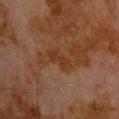Captured during whole-body skin photography for melanoma surveillance; the lesion was not biopsied.
The recorded lesion diameter is about 3 mm.
The subject is a male approximately 80 years of age.
Cropped from a total-body skin-imaging series; the visible field is about 15 mm.
Imaged with cross-polarized lighting.
Located on the upper back.
An algorithmic analysis of the crop reported a mean CIELAB color near L≈26 a*≈17 b*≈27 and a normalized lesion–skin contrast near 7.